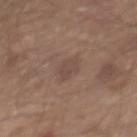No biopsy was performed on this lesion — it was imaged during a full skin examination and was not determined to be concerning. The lesion is located on the mid back. This image is a 15 mm lesion crop taken from a total-body photograph. Automated image analysis of the tile measured a mean CIELAB color near L≈46 a*≈16 b*≈23 and roughly 6 lightness units darker than nearby skin. Imaged with white-light lighting. A male subject aged 63 to 67.